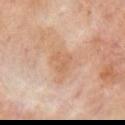The lesion was tiled from a total-body skin photograph and was not biopsied. A male subject aged around 70. Captured under cross-polarized illumination. Measured at roughly 2.5 mm in maximum diameter. An algorithmic analysis of the crop reported an eccentricity of roughly 0.55 and a symmetry-axis asymmetry near 0.45. The analysis additionally found border irregularity of about 4.5 on a 0–10 scale and a peripheral color-asymmetry measure near 0.5. A 15 mm crop from a total-body photograph taken for skin-cancer surveillance. Located on the arm.